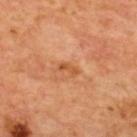Q: Was a biopsy performed?
A: imaged on a skin check; not biopsied
Q: Where on the body is the lesion?
A: the upper back
Q: What kind of image is this?
A: ~15 mm crop, total-body skin-cancer survey
Q: What are the patient's age and sex?
A: aged 63 to 67